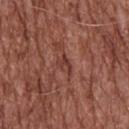This lesion was catalogued during total-body skin photography and was not selected for biopsy. Automated image analysis of the tile measured a nevus-likeness score of about 0/100. The recorded lesion diameter is about 2.5 mm. The lesion is located on the back. The subject is a male aged 73 to 77. A 15 mm close-up extracted from a 3D total-body photography capture. The tile uses white-light illumination.